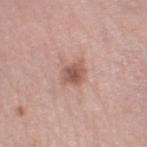Impression: Recorded during total-body skin imaging; not selected for excision or biopsy. Clinical summary: On the right lower leg. An algorithmic analysis of the crop reported a mean CIELAB color near L≈55 a*≈22 b*≈25 and about 12 CIELAB-L* units darker than the surrounding skin. The software also gave border irregularity of about 3 on a 0–10 scale and radial color variation of about 1. A roughly 15 mm field-of-view crop from a total-body skin photograph. The patient is a female aged 38–42. Captured under white-light illumination. About 2.5 mm across.